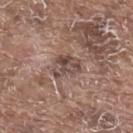Q: Automated lesion metrics?
A: an eccentricity of roughly 0.65 and two-axis asymmetry of about 0.35; a border-irregularity rating of about 4/10, a within-lesion color-variation index near 7/10, and peripheral color asymmetry of about 3; a nevus-likeness score of about 0/100 and a detector confidence of about 65 out of 100 that the crop contains a lesion
Q: Illumination type?
A: white-light illumination
Q: What is the imaging modality?
A: ~15 mm crop, total-body skin-cancer survey
Q: Where on the body is the lesion?
A: the upper back
Q: Who is the patient?
A: male, about 80 years old
Q: Lesion size?
A: ≈4 mm